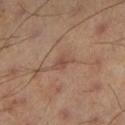{
  "biopsy_status": "not biopsied; imaged during a skin examination",
  "site": "left lower leg",
  "image": {
    "source": "total-body photography crop",
    "field_of_view_mm": 15
  },
  "lesion_size": {
    "long_diameter_mm_approx": 2.5
  },
  "patient": {
    "sex": "male",
    "age_approx": 45
  },
  "lighting": "cross-polarized"
}